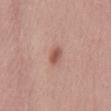Imaged with white-light lighting. Longest diameter approximately 3 mm. From the abdomen. A male patient in their mid-60s. A roughly 15 mm field-of-view crop from a total-body skin photograph.